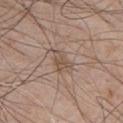<record>
  <biopsy_status>not biopsied; imaged during a skin examination</biopsy_status>
</record>